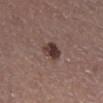notes=no biopsy performed (imaged during a skin exam)
illumination=white-light illumination
image-analysis metrics=a border-irregularity rating of about 2/10, internal color variation of about 4.5 on a 0–10 scale, and radial color variation of about 1.5; a nevus-likeness score of about 95/100 and lesion-presence confidence of about 100/100
location=the right lower leg
diameter=≈3 mm
imaging modality=total-body-photography crop, ~15 mm field of view
patient=male, aged 53 to 57